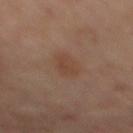Q: Is there a histopathology result?
A: catalogued during a skin exam; not biopsied
Q: What is the anatomic site?
A: the mid back
Q: What is the imaging modality?
A: 15 mm crop, total-body photography
Q: Who is the patient?
A: male, approximately 60 years of age